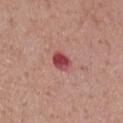Recorded during total-body skin imaging; not selected for excision or biopsy. A male subject approximately 70 years of age. From the chest. Captured under white-light illumination. This image is a 15 mm lesion crop taken from a total-body photograph. The recorded lesion diameter is about 2.5 mm.